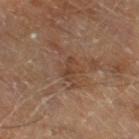Assessment:
Captured during whole-body skin photography for melanoma surveillance; the lesion was not biopsied.
Image and clinical context:
A 15 mm close-up tile from a total-body photography series done for melanoma screening. Approximately 3 mm at its widest. The subject is a male aged 68 to 72. The lesion is located on the right thigh. Captured under cross-polarized illumination.